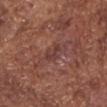Recorded during total-body skin imaging; not selected for excision or biopsy. This is a white-light tile. The lesion is located on the chest. About 3 mm across. Automated image analysis of the tile measured a symmetry-axis asymmetry near 0.5. The software also gave border irregularity of about 5.5 on a 0–10 scale and radial color variation of about 0. Cropped from a whole-body photographic skin survey; the tile spans about 15 mm. A male patient aged around 75.